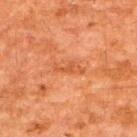Q: Is there a histopathology result?
A: catalogued during a skin exam; not biopsied
Q: How was this image acquired?
A: 15 mm crop, total-body photography
Q: Illumination type?
A: cross-polarized illumination
Q: What did automated image analysis measure?
A: a border-irregularity rating of about 6/10, internal color variation of about 0.5 on a 0–10 scale, and a peripheral color-asymmetry measure near 0; an automated nevus-likeness rating near 0 out of 100 and lesion-presence confidence of about 100/100
Q: What is the lesion's diameter?
A: ≈3.5 mm
Q: Patient demographics?
A: male, aged 58 to 62
Q: Where on the body is the lesion?
A: the upper back